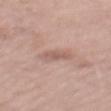No biopsy was performed on this lesion — it was imaged during a full skin examination and was not determined to be concerning.
The recorded lesion diameter is about 2.5 mm.
Captured under white-light illumination.
The lesion-visualizer software estimated a lesion color around L≈57 a*≈20 b*≈24 in CIELAB, a lesion–skin lightness drop of about 8, and a normalized border contrast of about 5.5. The software also gave internal color variation of about 1 on a 0–10 scale and radial color variation of about 0.5.
On the mid back.
A 15 mm close-up extracted from a 3D total-body photography capture.
A male subject, about 55 years old.